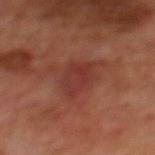follow-up = imaged on a skin check; not biopsied | diameter = ~3.5 mm (longest diameter) | tile lighting = cross-polarized | imaging modality = total-body-photography crop, ~15 mm field of view | patient = male, aged 68 to 72 | location = the mid back.